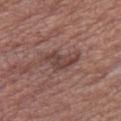Q: Was a biopsy performed?
A: catalogued during a skin exam; not biopsied
Q: How was this image acquired?
A: total-body-photography crop, ~15 mm field of view
Q: What is the anatomic site?
A: the right thigh
Q: Lesion size?
A: about 4.5 mm
Q: How was the tile lit?
A: white-light
Q: What are the patient's age and sex?
A: male, aged 73–77